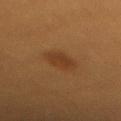Assessment:
Part of a total-body skin-imaging series; this lesion was reviewed on a skin check and was not flagged for biopsy.
Background:
From the mid back. Automated image analysis of the tile measured a footprint of about 6 mm², a shape eccentricity near 0.8, and a shape-asymmetry score of about 0.2 (0 = symmetric). It also reported an automated nevus-likeness rating near 100 out of 100. About 3.5 mm across. A female subject, roughly 30 years of age. A 15 mm close-up tile from a total-body photography series done for melanoma screening.